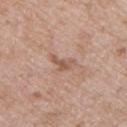Captured during whole-body skin photography for melanoma surveillance; the lesion was not biopsied.
A 15 mm close-up extracted from a 3D total-body photography capture.
On the chest.
A male patient in their 50s.